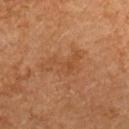Part of a total-body skin-imaging series; this lesion was reviewed on a skin check and was not flagged for biopsy.
On the upper back.
The lesion-visualizer software estimated a footprint of about 8 mm², a shape eccentricity near 0.85, and a shape-asymmetry score of about 0.55 (0 = symmetric). The analysis additionally found border irregularity of about 8 on a 0–10 scale, a color-variation rating of about 2/10, and radial color variation of about 0.5.
A 15 mm close-up extracted from a 3D total-body photography capture.
The recorded lesion diameter is about 5 mm.
A female patient approximately 60 years of age.